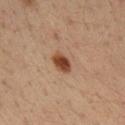follow-up: imaged on a skin check; not biopsied | size: ≈3 mm | body site: the right upper arm | imaging modality: total-body-photography crop, ~15 mm field of view | subject: male, aged approximately 35.